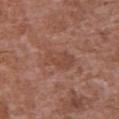notes = imaged on a skin check; not biopsied | subject = male, aged approximately 45 | imaging modality = ~15 mm crop, total-body skin-cancer survey | location = the chest.